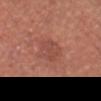Part of a total-body skin-imaging series; this lesion was reviewed on a skin check and was not flagged for biopsy.
The lesion is located on the head or neck.
A close-up tile cropped from a whole-body skin photograph, about 15 mm across.
The patient is a male aged approximately 45.
Imaged with white-light lighting.
The lesion-visualizer software estimated an eccentricity of roughly 0.65 and a shape-asymmetry score of about 0.15 (0 = symmetric). It also reported a mean CIELAB color near L≈48 a*≈26 b*≈27, a lesion–skin lightness drop of about 6, and a lesion-to-skin contrast of about 5 (normalized; higher = more distinct). It also reported a border-irregularity rating of about 2/10, a color-variation rating of about 3.5/10, and a peripheral color-asymmetry measure near 1.5.
Longest diameter approximately 4 mm.